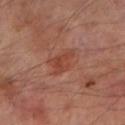Captured during whole-body skin photography for melanoma surveillance; the lesion was not biopsied.
The tile uses cross-polarized illumination.
The total-body-photography lesion software estimated an area of roughly 6 mm², an outline eccentricity of about 0.8 (0 = round, 1 = elongated), and a symmetry-axis asymmetry near 0.4. It also reported a mean CIELAB color near L≈42 a*≈26 b*≈29, about 7 CIELAB-L* units darker than the surrounding skin, and a lesion-to-skin contrast of about 6 (normalized; higher = more distinct). The software also gave an automated nevus-likeness rating near 25 out of 100.
Located on the left lower leg.
A roughly 15 mm field-of-view crop from a total-body skin photograph.
The subject is a male approximately 70 years of age.